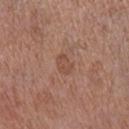Clinical impression:
Recorded during total-body skin imaging; not selected for excision or biopsy.
Clinical summary:
The subject is a female about 65 years old. The lesion is on the leg. A 15 mm crop from a total-body photograph taken for skin-cancer surveillance.